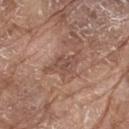Clinical impression:
No biopsy was performed on this lesion — it was imaged during a full skin examination and was not determined to be concerning.
Acquisition and patient details:
The tile uses white-light illumination. A male subject aged around 80. On the upper back. A lesion tile, about 15 mm wide, cut from a 3D total-body photograph. An algorithmic analysis of the crop reported a lesion area of about 7 mm², a shape eccentricity near 0.85, and a shape-asymmetry score of about 0.35 (0 = symmetric). It also reported a mean CIELAB color near L≈49 a*≈19 b*≈25, about 8 CIELAB-L* units darker than the surrounding skin, and a normalized lesion–skin contrast near 6. The software also gave border irregularity of about 5 on a 0–10 scale, a color-variation rating of about 2/10, and a peripheral color-asymmetry measure near 1. The software also gave an automated nevus-likeness rating near 0 out of 100 and a lesion-detection confidence of about 65/100. Longest diameter approximately 5 mm.